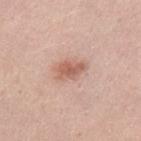Q: Is there a histopathology result?
A: no biopsy performed (imaged during a skin exam)
Q: Illumination type?
A: white-light
Q: What did automated image analysis measure?
A: a lesion area of about 5.5 mm², an outline eccentricity of about 0.8 (0 = round, 1 = elongated), and a symmetry-axis asymmetry near 0.25; a border-irregularity index near 2.5/10, internal color variation of about 3 on a 0–10 scale, and a peripheral color-asymmetry measure near 1; a nevus-likeness score of about 65/100 and a lesion-detection confidence of about 100/100
Q: Lesion size?
A: ≈3.5 mm
Q: Who is the patient?
A: male, in their 30s
Q: What is the imaging modality?
A: 15 mm crop, total-body photography